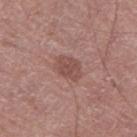Impression:
Captured during whole-body skin photography for melanoma surveillance; the lesion was not biopsied.
Clinical summary:
A male patient, aged 73–77. Automated image analysis of the tile measured two-axis asymmetry of about 0.25. It also reported a border-irregularity index near 2.5/10, internal color variation of about 2.5 on a 0–10 scale, and a peripheral color-asymmetry measure near 1. Captured under white-light illumination. Located on the left thigh. This image is a 15 mm lesion crop taken from a total-body photograph. The lesion's longest dimension is about 3.5 mm.